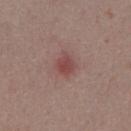Notes:
* follow-up: total-body-photography surveillance lesion; no biopsy
* lesion size: ~3 mm (longest diameter)
* patient: male, roughly 55 years of age
* location: the abdomen
* illumination: white-light illumination
* acquisition: ~15 mm crop, total-body skin-cancer survey
* automated metrics: an area of roughly 5 mm², a shape eccentricity near 0.65, and a shape-asymmetry score of about 0.2 (0 = symmetric); a lesion color around L≈46 a*≈23 b*≈21 in CIELAB, roughly 9 lightness units darker than nearby skin, and a lesion-to-skin contrast of about 7 (normalized; higher = more distinct); a border-irregularity rating of about 2/10, internal color variation of about 2 on a 0–10 scale, and a peripheral color-asymmetry measure near 0.5